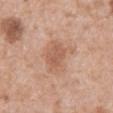Q: Was a biopsy performed?
A: total-body-photography surveillance lesion; no biopsy
Q: Lesion location?
A: the mid back
Q: What are the patient's age and sex?
A: male, aged 58–62
Q: How was the tile lit?
A: white-light illumination
Q: What is the lesion's diameter?
A: about 5 mm
Q: What kind of image is this?
A: ~15 mm tile from a whole-body skin photo